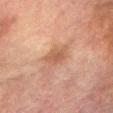About 3 mm across.
A region of skin cropped from a whole-body photographic capture, roughly 15 mm wide.
A male subject aged around 75.
Located on the right forearm.
An algorithmic analysis of the crop reported a lesion area of about 5 mm² and a shape eccentricity near 0.4. It also reported a mean CIELAB color near L≈47 a*≈19 b*≈28 and a normalized border contrast of about 6.